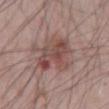follow-up = total-body-photography surveillance lesion; no biopsy
subject = male, about 65 years old
anatomic site = the abdomen
imaging modality = total-body-photography crop, ~15 mm field of view
diameter = ≈6 mm
tile lighting = white-light illumination